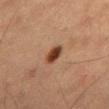Q: Was this lesion biopsied?
A: catalogued during a skin exam; not biopsied
Q: What is the lesion's diameter?
A: ≈2.5 mm
Q: What did automated image analysis measure?
A: a footprint of about 4 mm², a shape eccentricity near 0.7, and a shape-asymmetry score of about 0.2 (0 = symmetric); a normalized lesion–skin contrast near 11.5
Q: How was the tile lit?
A: cross-polarized illumination
Q: How was this image acquired?
A: total-body-photography crop, ~15 mm field of view
Q: Who is the patient?
A: male, aged around 60